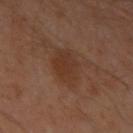size: ~4 mm (longest diameter); image source: ~15 mm tile from a whole-body skin photo; illumination: cross-polarized; anatomic site: the left upper arm; subject: male, aged 43 to 47.